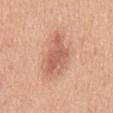biopsy_status: not biopsied; imaged during a skin examination
lesion_size:
  long_diameter_mm_approx: 6.5
automated_metrics:
  eccentricity: 0.85
  shape_asymmetry: 0.3
  border_irregularity_0_10: 3.5
  color_variation_0_10: 4.0
  peripheral_color_asymmetry: 1.5
site: mid back
lighting: white-light
image:
  source: total-body photography crop
  field_of_view_mm: 15
patient:
  sex: male
  age_approx: 50